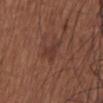Clinical impression:
The lesion was tiled from a total-body skin photograph and was not biopsied.
Image and clinical context:
Cropped from a whole-body photographic skin survey; the tile spans about 15 mm. The subject is a female in their mid- to late 70s. Located on the head or neck.